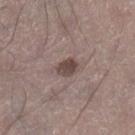Q: Was a biopsy performed?
A: imaged on a skin check; not biopsied
Q: How was the tile lit?
A: white-light illumination
Q: Automated lesion metrics?
A: border irregularity of about 1.5 on a 0–10 scale, a color-variation rating of about 3.5/10, and radial color variation of about 1.5; a nevus-likeness score of about 80/100
Q: How large is the lesion?
A: about 3 mm
Q: Lesion location?
A: the right lower leg
Q: How was this image acquired?
A: total-body-photography crop, ~15 mm field of view
Q: What are the patient's age and sex?
A: male, approximately 45 years of age